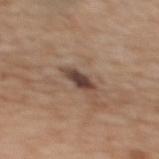Captured during whole-body skin photography for melanoma surveillance; the lesion was not biopsied. The lesion is on the upper back. A 15 mm crop from a total-body photograph taken for skin-cancer surveillance. A female patient aged 53 to 57.